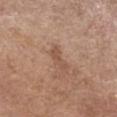Clinical impression: No biopsy was performed on this lesion — it was imaged during a full skin examination and was not determined to be concerning. Acquisition and patient details: The patient is a female about 65 years old. An algorithmic analysis of the crop reported about 7 CIELAB-L* units darker than the surrounding skin and a lesion-to-skin contrast of about 5.5 (normalized; higher = more distinct). And it measured a border-irregularity index near 5.5/10, a within-lesion color-variation index near 0/10, and peripheral color asymmetry of about 0. On the left forearm. This is a white-light tile. A roughly 15 mm field-of-view crop from a total-body skin photograph. The recorded lesion diameter is about 3.5 mm.